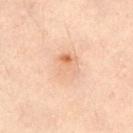Part of a total-body skin-imaging series; this lesion was reviewed on a skin check and was not flagged for biopsy.
The lesion is on the front of the torso.
An algorithmic analysis of the crop reported a mean CIELAB color near L≈60 a*≈17 b*≈30, roughly 6 lightness units darker than nearby skin, and a normalized border contrast of about 4.5. The software also gave a within-lesion color-variation index near 8/10 and a peripheral color-asymmetry measure near 2.5. And it measured a lesion-detection confidence of about 100/100.
A female patient about 55 years old.
The recorded lesion diameter is about 4 mm.
This is a cross-polarized tile.
A 15 mm close-up tile from a total-body photography series done for melanoma screening.